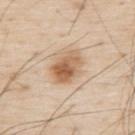Clinical impression: Recorded during total-body skin imaging; not selected for excision or biopsy. Clinical summary: A 15 mm close-up tile from a total-body photography series done for melanoma screening. On the upper back. The patient is a male approximately 80 years of age. Measured at roughly 4.5 mm in maximum diameter. Automated tile analysis of the lesion measured an average lesion color of about L≈62 a*≈18 b*≈34 (CIELAB), about 13 CIELAB-L* units darker than the surrounding skin, and a normalized border contrast of about 8.5. And it measured a border-irregularity rating of about 1.5/10, a within-lesion color-variation index near 7/10, and peripheral color asymmetry of about 2.5. The analysis additionally found a classifier nevus-likeness of about 95/100 and a detector confidence of about 100 out of 100 that the crop contains a lesion. This is a white-light tile.